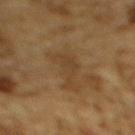The lesion was tiled from a total-body skin photograph and was not biopsied. The lesion-visualizer software estimated an average lesion color of about L≈35 a*≈13 b*≈29 (CIELAB) and a lesion–skin lightness drop of about 5. The analysis additionally found border irregularity of about 7.5 on a 0–10 scale and peripheral color asymmetry of about 0.5. The analysis additionally found an automated nevus-likeness rating near 0 out of 100 and a lesion-detection confidence of about 90/100. Captured under cross-polarized illumination. Longest diameter approximately 4.5 mm. A male subject aged approximately 85 or older. A 15 mm crop from a total-body photograph taken for skin-cancer surveillance. From the upper back.